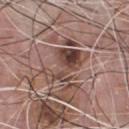biopsy status: total-body-photography surveillance lesion; no biopsy | patient: male, aged 73–77 | imaging modality: total-body-photography crop, ~15 mm field of view | lesion diameter: ~5.5 mm (longest diameter) | tile lighting: white-light illumination | location: the chest.